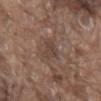Clinical impression: Part of a total-body skin-imaging series; this lesion was reviewed on a skin check and was not flagged for biopsy. Image and clinical context: Located on the mid back. This image is a 15 mm lesion crop taken from a total-body photograph. A male subject, about 80 years old.